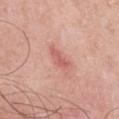Imaged during a routine full-body skin examination; the lesion was not biopsied and no histopathology is available. Imaged with white-light lighting. An algorithmic analysis of the crop reported a lesion area of about 4 mm², an outline eccentricity of about 0.9 (0 = round, 1 = elongated), and a symmetry-axis asymmetry near 0.35. And it measured a border-irregularity index near 4/10. The lesion is located on the chest. A 15 mm close-up extracted from a 3D total-body photography capture. Approximately 3.5 mm at its widest. The patient is a male aged around 55.